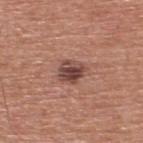The lesion was tiled from a total-body skin photograph and was not biopsied. Located on the upper back. A male subject, aged 53 to 57. A 15 mm close-up extracted from a 3D total-body photography capture.